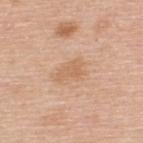This lesion was catalogued during total-body skin photography and was not selected for biopsy.
The patient is a male in their 50s.
An algorithmic analysis of the crop reported a footprint of about 5 mm², a shape eccentricity near 0.8, and a symmetry-axis asymmetry near 0.4. And it measured an average lesion color of about L≈63 a*≈20 b*≈35 (CIELAB), a lesion–skin lightness drop of about 7, and a lesion-to-skin contrast of about 5 (normalized; higher = more distinct). The analysis additionally found a border-irregularity rating of about 4.5/10 and internal color variation of about 1.5 on a 0–10 scale. And it measured a classifier nevus-likeness of about 0/100 and lesion-presence confidence of about 100/100.
Approximately 3.5 mm at its widest.
A 15 mm crop from a total-body photograph taken for skin-cancer surveillance.
Located on the upper back.